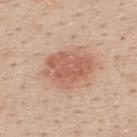Findings:
* notes — no biopsy performed (imaged during a skin exam)
* subject — male, aged approximately 30
* illumination — white-light
* site — the upper back
* diameter — about 5.5 mm
* image — 15 mm crop, total-body photography